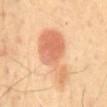| field | value |
|---|---|
| biopsy status | total-body-photography surveillance lesion; no biopsy |
| image | total-body-photography crop, ~15 mm field of view |
| subject | male, aged approximately 65 |
| site | the abdomen |